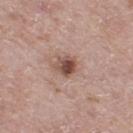Imaged during a routine full-body skin examination; the lesion was not biopsied and no histopathology is available.
The lesion-visualizer software estimated an average lesion color of about L≈49 a*≈20 b*≈24 (CIELAB), a lesion–skin lightness drop of about 14, and a normalized lesion–skin contrast near 10. It also reported a border-irregularity rating of about 1.5/10, a within-lesion color-variation index near 5/10, and a peripheral color-asymmetry measure near 1.5. The software also gave a classifier nevus-likeness of about 55/100 and a lesion-detection confidence of about 100/100.
On the left thigh.
Imaged with white-light lighting.
A male patient, in their 60s.
Cropped from a total-body skin-imaging series; the visible field is about 15 mm.